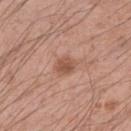Background: From the left upper arm. A male patient aged 43–47. A lesion tile, about 15 mm wide, cut from a 3D total-body photograph.